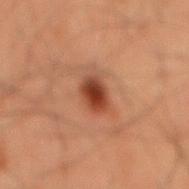follow-up: no biopsy performed (imaged during a skin exam) | automated lesion analysis: an average lesion color of about L≈32 a*≈22 b*≈27 (CIELAB), roughly 12 lightness units darker than nearby skin, and a normalized border contrast of about 11; border irregularity of about 2.5 on a 0–10 scale, a within-lesion color-variation index near 4.5/10, and peripheral color asymmetry of about 1.5 | size: ≈3 mm | body site: the mid back | acquisition: total-body-photography crop, ~15 mm field of view | patient: male, aged 58–62 | illumination: cross-polarized.